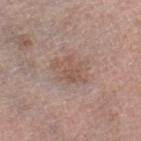biopsy status: no biopsy performed (imaged during a skin exam); anatomic site: the right lower leg; subject: female, in their 70s; acquisition: ~15 mm crop, total-body skin-cancer survey; lesion size: about 4.5 mm; illumination: white-light.